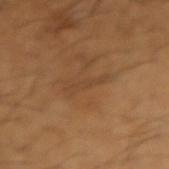| feature | finding |
|---|---|
| follow-up | no biopsy performed (imaged during a skin exam) |